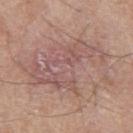<tbp_lesion>
  <biopsy_status>not biopsied; imaged during a skin examination</biopsy_status>
  <patient>
    <sex>male</sex>
    <age_approx>80</age_approx>
  </patient>
  <image>
    <source>total-body photography crop</source>
    <field_of_view_mm>15</field_of_view_mm>
  </image>
  <site>right thigh</site>
  <lighting>white-light</lighting>
</tbp_lesion>